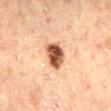- notes · total-body-photography surveillance lesion; no biopsy
- anatomic site · the mid back
- lesion diameter · ≈3.5 mm
- acquisition · ~15 mm tile from a whole-body skin photo
- tile lighting · cross-polarized illumination
- patient · male, aged around 50